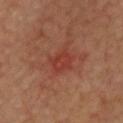  biopsy_status: not biopsied; imaged during a skin examination
  lighting: cross-polarized
  patient:
    sex: male
    age_approx: 60
  automated_metrics:
    area_mm2_approx: 5.5
    eccentricity: 0.65
    shape_asymmetry: 0.25
    cielab_L: 38
    cielab_a: 30
    cielab_b: 29
    vs_skin_darker_L: 6.0
    border_irregularity_0_10: 2.5
    color_variation_0_10: 2.0
    peripheral_color_asymmetry: 1.0
    nevus_likeness_0_100: 5
    lesion_detection_confidence_0_100: 100
  image:
    source: total-body photography crop
    field_of_view_mm: 15
  site: chest